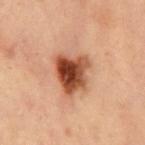Clinical impression: No biopsy was performed on this lesion — it was imaged during a full skin examination and was not determined to be concerning. Acquisition and patient details: About 4.5 mm across. Cropped from a total-body skin-imaging series; the visible field is about 15 mm. Automated image analysis of the tile measured a lesion area of about 13 mm². The software also gave a lesion color around L≈38 a*≈22 b*≈29 in CIELAB and a lesion-to-skin contrast of about 13 (normalized; higher = more distinct). And it measured a nevus-likeness score of about 100/100 and a lesion-detection confidence of about 100/100. The lesion is on the chest. The tile uses cross-polarized illumination. The subject is a male approximately 55 years of age.